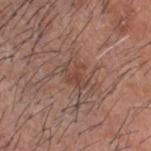Impression:
The lesion was tiled from a total-body skin photograph and was not biopsied.
Context:
A male patient aged 53–57. Approximately 3 mm at its widest. A 15 mm close-up tile from a total-body photography series done for melanoma screening. Imaged with white-light lighting. On the head or neck.